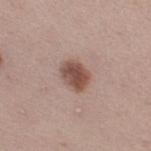<lesion>
  <biopsy_status>not biopsied; imaged during a skin examination</biopsy_status>
  <site>right thigh</site>
  <patient>
    <sex>female</sex>
    <age_approx>45</age_approx>
  </patient>
  <automated_metrics>
    <area_mm2_approx>7.5</area_mm2_approx>
    <eccentricity>0.65</eccentricity>
    <shape_asymmetry>0.2</shape_asymmetry>
    <cielab_L>50</cielab_L>
    <cielab_a>20</cielab_a>
    <cielab_b>24</cielab_b>
    <vs_skin_darker_L>13.0</vs_skin_darker_L>
    <vs_skin_contrast_norm>9.5</vs_skin_contrast_norm>
    <border_irregularity_0_10>2.0</border_irregularity_0_10>
    <color_variation_0_10>3.5</color_variation_0_10>
    <lesion_detection_confidence_0_100>100</lesion_detection_confidence_0_100>
  </automated_metrics>
  <image>
    <source>total-body photography crop</source>
    <field_of_view_mm>15</field_of_view_mm>
  </image>
  <lighting>white-light</lighting>
  <lesion_size>
    <long_diameter_mm_approx>3.5</long_diameter_mm_approx>
  </lesion_size>
</lesion>